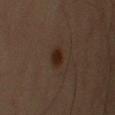This lesion was catalogued during total-body skin photography and was not selected for biopsy.
A male subject aged 53 to 57.
A roughly 15 mm field-of-view crop from a total-body skin photograph.
Automated image analysis of the tile measured an average lesion color of about L≈23 a*≈15 b*≈22 (CIELAB), a lesion–skin lightness drop of about 7, and a lesion-to-skin contrast of about 9 (normalized; higher = more distinct). The software also gave border irregularity of about 1.5 on a 0–10 scale, a color-variation rating of about 3.5/10, and a peripheral color-asymmetry measure near 1.5. The analysis additionally found lesion-presence confidence of about 100/100.
Located on the right upper arm.
The tile uses cross-polarized illumination.
Longest diameter approximately 2.5 mm.